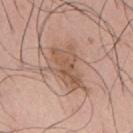anatomic site = the mid back
subject = male, approximately 40 years of age
TBP lesion metrics = a lesion area of about 14 mm² and two-axis asymmetry of about 0.4; a lesion color around L≈56 a*≈19 b*≈29 in CIELAB, a lesion–skin lightness drop of about 9, and a normalized lesion–skin contrast near 7; border irregularity of about 4.5 on a 0–10 scale, internal color variation of about 4.5 on a 0–10 scale, and peripheral color asymmetry of about 1.5
image = total-body-photography crop, ~15 mm field of view
lesion diameter = about 6 mm